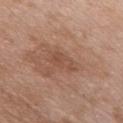The lesion was tiled from a total-body skin photograph and was not biopsied. From the chest. A male subject aged around 50. A close-up tile cropped from a whole-body skin photograph, about 15 mm across.